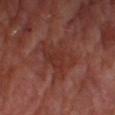Q: Was a biopsy performed?
A: no biopsy performed (imaged during a skin exam)
Q: Automated lesion metrics?
A: an area of roughly 14 mm² and a symmetry-axis asymmetry near 0.5; a border-irregularity index near 6.5/10 and a within-lesion color-variation index near 2.5/10
Q: What is the anatomic site?
A: the right lower leg
Q: What is the lesion's diameter?
A: about 5 mm
Q: Who is the patient?
A: male, approximately 70 years of age
Q: What lighting was used for the tile?
A: cross-polarized
Q: What kind of image is this?
A: 15 mm crop, total-body photography